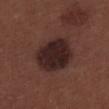Case summary:
– biopsy status — no biopsy performed (imaged during a skin exam)
– acquisition — ~15 mm tile from a whole-body skin photo
– lesion size — ≈5.5 mm
– site — the upper back
– subject — male, aged 28–32